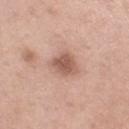No biopsy was performed on this lesion — it was imaged during a full skin examination and was not determined to be concerning. A female subject, about 55 years old. The lesion is on the leg. The tile uses white-light illumination. A 15 mm close-up extracted from a 3D total-body photography capture. Approximately 3 mm at its widest.